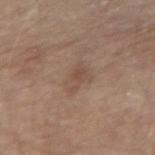Assessment: Captured during whole-body skin photography for melanoma surveillance; the lesion was not biopsied. Image and clinical context: This is a white-light tile. A male subject approximately 70 years of age. A region of skin cropped from a whole-body photographic capture, roughly 15 mm wide. Automated image analysis of the tile measured an area of roughly 5.5 mm², an eccentricity of roughly 0.8, and a shape-asymmetry score of about 0.35 (0 = symmetric). The software also gave an automated nevus-likeness rating near 0 out of 100. The lesion is located on the left forearm.